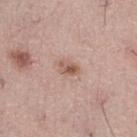Q: Was a biopsy performed?
A: total-body-photography surveillance lesion; no biopsy
Q: Patient demographics?
A: male, in their mid-50s
Q: What kind of image is this?
A: ~15 mm tile from a whole-body skin photo